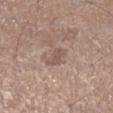Q: What did automated image analysis measure?
A: a mean CIELAB color near L≈53 a*≈16 b*≈23, a lesion–skin lightness drop of about 8, and a normalized border contrast of about 5.5
Q: Lesion location?
A: the right lower leg
Q: How was the tile lit?
A: white-light
Q: Who is the patient?
A: male, about 60 years old
Q: How large is the lesion?
A: ≈3 mm
Q: What kind of image is this?
A: ~15 mm tile from a whole-body skin photo